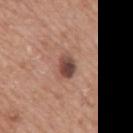workup: imaged on a skin check; not biopsied | acquisition: total-body-photography crop, ~15 mm field of view | body site: the mid back | lighting: white-light illumination | patient: male, roughly 75 years of age | automated metrics: a mean CIELAB color near L≈46 a*≈21 b*≈24, about 14 CIELAB-L* units darker than the surrounding skin, and a lesion-to-skin contrast of about 10.5 (normalized; higher = more distinct); an automated nevus-likeness rating near 60 out of 100 and a detector confidence of about 100 out of 100 that the crop contains a lesion.